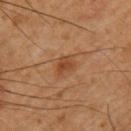Q: Was a biopsy performed?
A: catalogued during a skin exam; not biopsied
Q: Automated lesion metrics?
A: a color-variation rating of about 2.5/10 and a peripheral color-asymmetry measure near 1
Q: Where on the body is the lesion?
A: the right upper arm
Q: What kind of image is this?
A: 15 mm crop, total-body photography
Q: What are the patient's age and sex?
A: male, aged 58–62